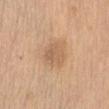- notes — imaged on a skin check; not biopsied
- patient — female, aged 63 to 67
- illumination — white-light
- anatomic site — the abdomen
- image — total-body-photography crop, ~15 mm field of view